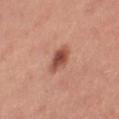anatomic site: the leg | subject: female, roughly 25 years of age | image-analysis metrics: a lesion area of about 6.5 mm², an outline eccentricity of about 0.85 (0 = round, 1 = elongated), and a shape-asymmetry score of about 0.2 (0 = symmetric); an average lesion color of about L≈51 a*≈26 b*≈30 (CIELAB), about 13 CIELAB-L* units darker than the surrounding skin, and a normalized lesion–skin contrast near 9; a border-irregularity index near 2/10 and a peripheral color-asymmetry measure near 1.5; a nevus-likeness score of about 90/100 and a detector confidence of about 100 out of 100 that the crop contains a lesion | acquisition: ~15 mm crop, total-body skin-cancer survey.